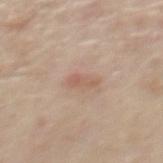Recorded during total-body skin imaging; not selected for excision or biopsy. The subject is a male in their mid- to late 50s. Measured at roughly 3 mm in maximum diameter. Automated tile analysis of the lesion measured an area of roughly 2.5 mm² and an outline eccentricity of about 0.95 (0 = round, 1 = elongated). The analysis additionally found a within-lesion color-variation index near 0/10 and radial color variation of about 0. Cropped from a whole-body photographic skin survey; the tile spans about 15 mm. The tile uses white-light illumination. Located on the mid back.